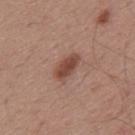Recorded during total-body skin imaging; not selected for excision or biopsy. Captured under white-light illumination. On the upper back. A male subject, in their mid-50s. A close-up tile cropped from a whole-body skin photograph, about 15 mm across. Longest diameter approximately 4 mm.